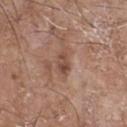<tbp_lesion>
  <automated_metrics>
    <area_mm2_approx>4.0</area_mm2_approx>
    <shape_asymmetry>0.2</shape_asymmetry>
    <border_irregularity_0_10>2.0</border_irregularity_0_10>
    <color_variation_0_10>3.5</color_variation_0_10>
    <peripheral_color_asymmetry>1.5</peripheral_color_asymmetry>
  </automated_metrics>
  <lighting>white-light</lighting>
  <patient>
    <sex>male</sex>
    <age_approx>70</age_approx>
  </patient>
  <site>chest</site>
  <lesion_size>
    <long_diameter_mm_approx>2.5</long_diameter_mm_approx>
  </lesion_size>
  <image>
    <source>total-body photography crop</source>
    <field_of_view_mm>15</field_of_view_mm>
  </image>
</tbp_lesion>